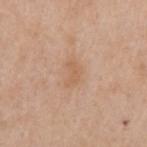Q: Was this lesion biopsied?
A: catalogued during a skin exam; not biopsied
Q: What lighting was used for the tile?
A: white-light
Q: What kind of image is this?
A: total-body-photography crop, ~15 mm field of view
Q: How large is the lesion?
A: ≈2.5 mm
Q: Lesion location?
A: the left upper arm
Q: Who is the patient?
A: male, aged around 60